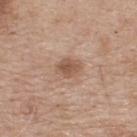workup: no biopsy performed (imaged during a skin exam) | lesion diameter: ~3 mm (longest diameter) | automated metrics: an area of roughly 5.5 mm², an eccentricity of roughly 0.7, and a symmetry-axis asymmetry near 0.15; an average lesion color of about L≈54 a*≈19 b*≈29 (CIELAB) and roughly 10 lightness units darker than nearby skin | site: the upper back | acquisition: ~15 mm crop, total-body skin-cancer survey | lighting: white-light illumination | subject: male, approximately 75 years of age.